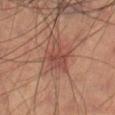Recorded during total-body skin imaging; not selected for excision or biopsy. A male subject aged approximately 70. Approximately 4 mm at its widest. The lesion is located on the right thigh. Cropped from a whole-body photographic skin survey; the tile spans about 15 mm.